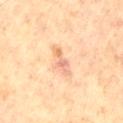Recorded during total-body skin imaging; not selected for excision or biopsy. Approximately 3.5 mm at its widest. An algorithmic analysis of the crop reported an average lesion color of about L≈72 a*≈23 b*≈35 (CIELAB) and a normalized border contrast of about 5.5. Captured under cross-polarized illumination. A region of skin cropped from a whole-body photographic capture, roughly 15 mm wide. A male patient, about 65 years old.